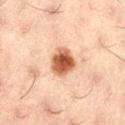Case summary:
• workup · no biopsy performed (imaged during a skin exam)